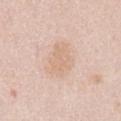biopsy status=catalogued during a skin exam; not biopsied | patient=female, aged 63 to 67 | location=the front of the torso | illumination=white-light | image=total-body-photography crop, ~15 mm field of view | diameter=≈4.5 mm.